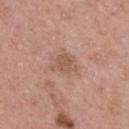<record>
  <biopsy_status>not biopsied; imaged during a skin examination</biopsy_status>
  <image>
    <source>total-body photography crop</source>
    <field_of_view_mm>15</field_of_view_mm>
  </image>
  <lesion_size>
    <long_diameter_mm_approx>3.5</long_diameter_mm_approx>
  </lesion_size>
  <lighting>white-light</lighting>
  <site>upper back</site>
  <patient>
    <sex>male</sex>
    <age_approx>65</age_approx>
  </patient>
</record>